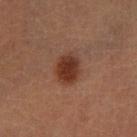<lesion>
  <biopsy_status>not biopsied; imaged during a skin examination</biopsy_status>
  <automated_metrics>
    <border_irregularity_0_10>1.5</border_irregularity_0_10>
    <color_variation_0_10>3.0</color_variation_0_10>
    <peripheral_color_asymmetry>1.0</peripheral_color_asymmetry>
    <nevus_likeness_0_100>100</nevus_likeness_0_100>
    <lesion_detection_confidence_0_100>100</lesion_detection_confidence_0_100>
  </automated_metrics>
  <lesion_size>
    <long_diameter_mm_approx>3.5</long_diameter_mm_approx>
  </lesion_size>
  <site>left lower leg</site>
  <image>
    <source>total-body photography crop</source>
    <field_of_view_mm>15</field_of_view_mm>
  </image>
  <lighting>cross-polarized</lighting>
  <patient>
    <sex>male</sex>
    <age_approx>60</age_approx>
  </patient>
</lesion>